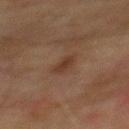No biopsy was performed on this lesion — it was imaged during a full skin examination and was not determined to be concerning.
The patient is a male aged 73–77.
About 3 mm across.
The lesion is located on the back.
Cropped from a whole-body photographic skin survey; the tile spans about 15 mm.
The tile uses cross-polarized illumination.